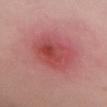{
  "biopsy_status": "not biopsied; imaged during a skin examination",
  "site": "chest",
  "patient": {
    "sex": "female",
    "age_approx": 25
  },
  "lesion_size": {
    "long_diameter_mm_approx": 5.5
  },
  "image": {
    "source": "total-body photography crop",
    "field_of_view_mm": 15
  },
  "automated_metrics": {
    "cielab_L": 49,
    "cielab_a": 34,
    "cielab_b": 25,
    "vs_skin_contrast_norm": 7.0
  }
}